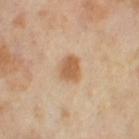biopsy status = catalogued during a skin exam; not biopsied | image source = ~15 mm tile from a whole-body skin photo | TBP lesion metrics = a lesion area of about 6.5 mm² and an eccentricity of roughly 0.55; a border-irregularity index near 2/10, internal color variation of about 3 on a 0–10 scale, and a peripheral color-asymmetry measure near 1; a nevus-likeness score of about 100/100 and a lesion-detection confidence of about 100/100 | site = the left thigh | lesion size = ≈3 mm | patient = female, aged around 50 | lighting = cross-polarized illumination.